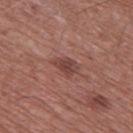| feature | finding |
|---|---|
| subject | male, aged approximately 60 |
| diameter | ≈3 mm |
| image source | 15 mm crop, total-body photography |
| body site | the right thigh |
| illumination | white-light |